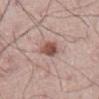Clinical impression: Recorded during total-body skin imaging; not selected for excision or biopsy. Image and clinical context: A male subject aged approximately 65. The lesion's longest dimension is about 3 mm. The tile uses white-light illumination. The lesion is located on the abdomen. A lesion tile, about 15 mm wide, cut from a 3D total-body photograph.